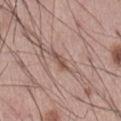{"biopsy_status": "not biopsied; imaged during a skin examination", "image": {"source": "total-body photography crop", "field_of_view_mm": 15}, "lesion_size": {"long_diameter_mm_approx": 2.5}, "site": "abdomen", "patient": {"sex": "male", "age_approx": 55}}